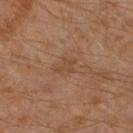This lesion was catalogued during total-body skin photography and was not selected for biopsy. Located on the right lower leg. About 2.5 mm across. This is a cross-polarized tile. Cropped from a whole-body photographic skin survey; the tile spans about 15 mm. The subject is a male aged 58–62. Automated image analysis of the tile measured an area of roughly 3 mm² and a symmetry-axis asymmetry near 0.4. The analysis additionally found a border-irregularity index near 4.5/10, internal color variation of about 0 on a 0–10 scale, and peripheral color asymmetry of about 0.